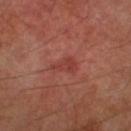biopsy status = catalogued during a skin exam; not biopsied.